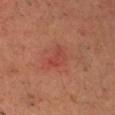Q: Was a biopsy performed?
A: no biopsy performed (imaged during a skin exam)
Q: What kind of image is this?
A: 15 mm crop, total-body photography
Q: What is the anatomic site?
A: the chest
Q: What are the patient's age and sex?
A: male, aged 58–62
Q: What did automated image analysis measure?
A: a lesion color around L≈42 a*≈29 b*≈28 in CIELAB, about 6 CIELAB-L* units darker than the surrounding skin, and a normalized lesion–skin contrast near 4.5; an automated nevus-likeness rating near 0 out of 100 and a lesion-detection confidence of about 100/100